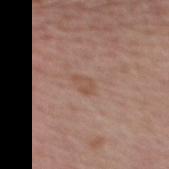A region of skin cropped from a whole-body photographic capture, roughly 15 mm wide. From the upper back. A female patient, in their mid-60s. Imaged with white-light lighting.